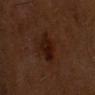notes: total-body-photography surveillance lesion; no biopsy.